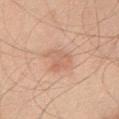{
  "lighting": "white-light",
  "lesion_size": {
    "long_diameter_mm_approx": 3.5
  },
  "image": {
    "source": "total-body photography crop",
    "field_of_view_mm": 15
  },
  "site": "chest",
  "patient": {
    "sex": "male",
    "age_approx": 45
  }
}